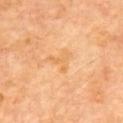{
  "biopsy_status": "not biopsied; imaged during a skin examination",
  "lesion_size": {
    "long_diameter_mm_approx": 3.0
  },
  "site": "upper back",
  "lighting": "cross-polarized",
  "patient": {
    "sex": "male",
    "age_approx": 60
  },
  "image": {
    "source": "total-body photography crop",
    "field_of_view_mm": 15
  }
}